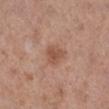Notes:
– follow-up: catalogued during a skin exam; not biopsied
– imaging modality: total-body-photography crop, ~15 mm field of view
– subject: female, aged approximately 40
– location: the leg
– illumination: white-light illumination
– diameter: ~3 mm (longest diameter)